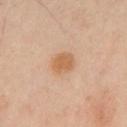Assessment: Part of a total-body skin-imaging series; this lesion was reviewed on a skin check and was not flagged for biopsy. Image and clinical context: A male subject aged 63 to 67. From the chest. About 3 mm across. A roughly 15 mm field-of-view crop from a total-body skin photograph. Captured under cross-polarized illumination.